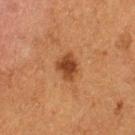Q: Was a biopsy performed?
A: catalogued during a skin exam; not biopsied
Q: What is the anatomic site?
A: the leg
Q: What is the lesion's diameter?
A: ~3 mm (longest diameter)
Q: How was this image acquired?
A: 15 mm crop, total-body photography
Q: Who is the patient?
A: female, aged 48 to 52
Q: What lighting was used for the tile?
A: cross-polarized illumination